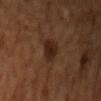The lesion was photographed on a routine skin check and not biopsied; there is no pathology result. The tile uses cross-polarized illumination. Located on the head or neck. A 15 mm close-up tile from a total-body photography series done for melanoma screening. The lesion-visualizer software estimated a border-irregularity index near 2/10 and peripheral color asymmetry of about 0.5. Measured at roughly 3.5 mm in maximum diameter. A male patient, aged approximately 55.